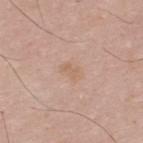{"site": "back", "patient": {"sex": "male", "age_approx": 40}, "lighting": "white-light", "lesion_size": {"long_diameter_mm_approx": 2.5}, "image": {"source": "total-body photography crop", "field_of_view_mm": 15}}